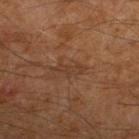  biopsy_status: not biopsied; imaged during a skin examination
  lighting: cross-polarized
  image:
    source: total-body photography crop
    field_of_view_mm: 15
  lesion_size:
    long_diameter_mm_approx: 3.5
  site: right lower leg
  patient:
    sex: male
    age_approx: 60
  automated_metrics:
    area_mm2_approx: 4.0
    eccentricity: 0.9
    shape_asymmetry: 0.35
    border_irregularity_0_10: 5.0
    color_variation_0_10: 0.5
    peripheral_color_asymmetry: 0.0
    lesion_detection_confidence_0_100: 95